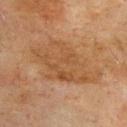Clinical impression:
This lesion was catalogued during total-body skin photography and was not selected for biopsy.
Acquisition and patient details:
Imaged with cross-polarized lighting. From the upper back. The patient is a male in their mid- to late 70s. Cropped from a total-body skin-imaging series; the visible field is about 15 mm.